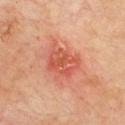Clinical impression:
No biopsy was performed on this lesion — it was imaged during a full skin examination and was not determined to be concerning.
Context:
The recorded lesion diameter is about 4 mm. A male patient, approximately 70 years of age. Automated tile analysis of the lesion measured a footprint of about 11 mm², a shape eccentricity near 0.65, and two-axis asymmetry of about 0.2. The analysis additionally found radial color variation of about 2. This is a cross-polarized tile. Located on the chest. Cropped from a whole-body photographic skin survey; the tile spans about 15 mm.